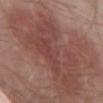{"biopsy_status": "not biopsied; imaged during a skin examination", "image": {"source": "total-body photography crop", "field_of_view_mm": 15}, "patient": {"sex": "male", "age_approx": 55}, "lesion_size": {"long_diameter_mm_approx": 15.0}, "site": "abdomen", "lighting": "white-light"}